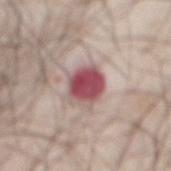The lesion was photographed on a routine skin check and not biopsied; there is no pathology result.
The patient is a male roughly 70 years of age.
From the front of the torso.
A 15 mm close-up tile from a total-body photography series done for melanoma screening.